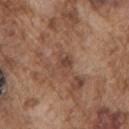Assessment:
Imaged during a routine full-body skin examination; the lesion was not biopsied and no histopathology is available.
Acquisition and patient details:
This image is a 15 mm lesion crop taken from a total-body photograph. A male subject in their mid- to late 70s. From the left upper arm.